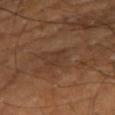{
  "biopsy_status": "not biopsied; imaged during a skin examination",
  "automated_metrics": {
    "eccentricity": 0.95,
    "cielab_L": 32,
    "cielab_a": 17,
    "cielab_b": 25,
    "vs_skin_darker_L": 5.0,
    "vs_skin_contrast_norm": 5.0,
    "border_irregularity_0_10": 4.0,
    "peripheral_color_asymmetry": 0.0,
    "nevus_likeness_0_100": 0,
    "lesion_detection_confidence_0_100": 100
  },
  "image": {
    "source": "total-body photography crop",
    "field_of_view_mm": 15
  },
  "site": "right arm",
  "lighting": "cross-polarized",
  "lesion_size": {
    "long_diameter_mm_approx": 2.5
  },
  "patient": {
    "sex": "male",
    "age_approx": 60
  }
}